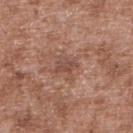| key | value |
|---|---|
| workup | total-body-photography surveillance lesion; no biopsy |
| image source | ~15 mm crop, total-body skin-cancer survey |
| subject | male, aged approximately 45 |
| site | the upper back |